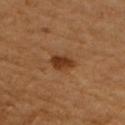Q: Is there a histopathology result?
A: imaged on a skin check; not biopsied
Q: Lesion location?
A: the right upper arm
Q: How was the tile lit?
A: cross-polarized
Q: What kind of image is this?
A: ~15 mm crop, total-body skin-cancer survey
Q: How large is the lesion?
A: ≈2.5 mm
Q: Patient demographics?
A: female, in their mid-50s